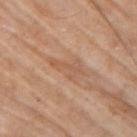The lesion was photographed on a routine skin check and not biopsied; there is no pathology result.
The tile uses white-light illumination.
The recorded lesion diameter is about 4 mm.
A roughly 15 mm field-of-view crop from a total-body skin photograph.
On the right upper arm.
The subject is a male approximately 80 years of age.